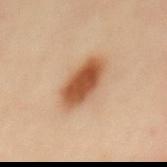Part of a total-body skin-imaging series; this lesion was reviewed on a skin check and was not flagged for biopsy. Longest diameter approximately 5.5 mm. The lesion is located on the mid back. This image is a 15 mm lesion crop taken from a total-body photograph. The subject is a female in their mid- to late 30s.